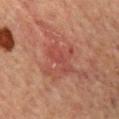Assessment:
Recorded during total-body skin imaging; not selected for excision or biopsy.
Image and clinical context:
Longest diameter approximately 4.5 mm. The patient is a male in their mid-60s. From the mid back. The total-body-photography lesion software estimated a lesion area of about 6.5 mm² and two-axis asymmetry of about 0.3. The software also gave an average lesion color of about L≈42 a*≈27 b*≈26 (CIELAB), roughly 6 lightness units darker than nearby skin, and a lesion-to-skin contrast of about 5 (normalized; higher = more distinct). A close-up tile cropped from a whole-body skin photograph, about 15 mm across.